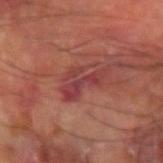{
  "lesion_size": {
    "long_diameter_mm_approx": 5.5
  },
  "patient": {
    "sex": "male",
    "age_approx": 60
  },
  "site": "right arm",
  "automated_metrics": {
    "area_mm2_approx": 8.0,
    "eccentricity": 0.85,
    "shape_asymmetry": 0.65
  },
  "image": {
    "source": "total-body photography crop",
    "field_of_view_mm": 15
  }
}